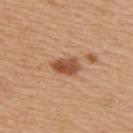| feature | finding |
|---|---|
| biopsy status | no biopsy performed (imaged during a skin exam) |
| TBP lesion metrics | an average lesion color of about L≈51 a*≈23 b*≈36 (CIELAB) and about 13 CIELAB-L* units darker than the surrounding skin; border irregularity of about 2.5 on a 0–10 scale, internal color variation of about 4 on a 0–10 scale, and peripheral color asymmetry of about 1.5 |
| subject | male, in their mid- to late 60s |
| anatomic site | the upper back |
| lesion diameter | ≈3.5 mm |
| image | ~15 mm crop, total-body skin-cancer survey |